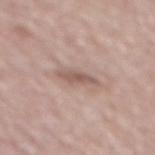{
  "site": "mid back",
  "patient": {
    "sex": "male",
    "age_approx": 80
  },
  "image": {
    "source": "total-body photography crop",
    "field_of_view_mm": 15
  },
  "lighting": "white-light",
  "lesion_size": {
    "long_diameter_mm_approx": 3.5
  }
}